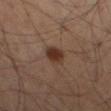{
  "biopsy_status": "not biopsied; imaged during a skin examination",
  "site": "left thigh",
  "lesion_size": {
    "long_diameter_mm_approx": 3.0
  },
  "image": {
    "source": "total-body photography crop",
    "field_of_view_mm": 15
  },
  "lighting": "cross-polarized",
  "automated_metrics": {
    "area_mm2_approx": 5.5,
    "shape_asymmetry": 0.15,
    "nevus_likeness_0_100": 95,
    "lesion_detection_confidence_0_100": 100
  },
  "patient": {
    "sex": "male",
    "age_approx": 65
  }
}